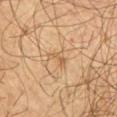<case>
  <biopsy_status>not biopsied; imaged during a skin examination</biopsy_status>
  <patient>
    <sex>male</sex>
    <age_approx>30</age_approx>
  </patient>
  <site>chest</site>
  <image>
    <source>total-body photography crop</source>
    <field_of_view_mm>15</field_of_view_mm>
  </image>
</case>